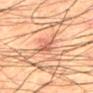<tbp_lesion>
<biopsy_status>not biopsied; imaged during a skin examination</biopsy_status>
<image>
  <source>total-body photography crop</source>
  <field_of_view_mm>15</field_of_view_mm>
</image>
<lesion_size>
  <long_diameter_mm_approx>3.5</long_diameter_mm_approx>
</lesion_size>
<site>mid back</site>
<lighting>cross-polarized</lighting>
<patient>
  <sex>male</sex>
  <age_approx>65</age_approx>
</patient>
</tbp_lesion>